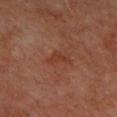A lesion tile, about 15 mm wide, cut from a 3D total-body photograph. Located on the upper back. Measured at roughly 2.5 mm in maximum diameter. This is a cross-polarized tile. An algorithmic analysis of the crop reported a lesion area of about 2.5 mm², an outline eccentricity of about 0.9 (0 = round, 1 = elongated), and two-axis asymmetry of about 0.5. The software also gave an average lesion color of about L≈33 a*≈23 b*≈28 (CIELAB), about 5 CIELAB-L* units darker than the surrounding skin, and a normalized lesion–skin contrast near 6. The analysis additionally found border irregularity of about 5 on a 0–10 scale, internal color variation of about 0 on a 0–10 scale, and peripheral color asymmetry of about 0. And it measured a classifier nevus-likeness of about 0/100 and lesion-presence confidence of about 100/100. A male patient, aged 58 to 62.